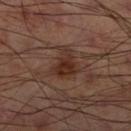Clinical impression:
The lesion was photographed on a routine skin check and not biopsied; there is no pathology result.
Clinical summary:
Cropped from a whole-body photographic skin survey; the tile spans about 15 mm. From the right lower leg. The lesion's longest dimension is about 4 mm.